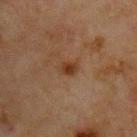Q: Was a biopsy performed?
A: total-body-photography surveillance lesion; no biopsy
Q: Lesion size?
A: about 2.5 mm
Q: Where on the body is the lesion?
A: the chest
Q: Patient demographics?
A: male, in their mid- to late 60s
Q: What is the imaging modality?
A: ~15 mm tile from a whole-body skin photo